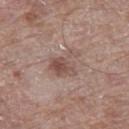{"biopsy_status": "not biopsied; imaged during a skin examination"}